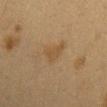Imaged during a routine full-body skin examination; the lesion was not biopsied and no histopathology is available.
The lesion's longest dimension is about 3 mm.
A 15 mm crop from a total-body photograph taken for skin-cancer surveillance.
A female patient about 40 years old.
This is a cross-polarized tile.
Automated tile analysis of the lesion measured border irregularity of about 3.5 on a 0–10 scale and a color-variation rating of about 2/10. And it measured a classifier nevus-likeness of about 5/100 and lesion-presence confidence of about 100/100.
Located on the mid back.